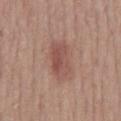Findings:
- notes: catalogued during a skin exam; not biopsied
- diameter: ≈4.5 mm
- body site: the mid back
- imaging modality: ~15 mm tile from a whole-body skin photo
- subject: male, in their 60s